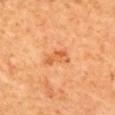Findings:
* body site · the upper back
* patient · female
* imaging modality · 15 mm crop, total-body photography
* lesion size · about 3.5 mm
* tile lighting · cross-polarized